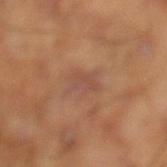{
  "biopsy_status": "not biopsied; imaged during a skin examination",
  "site": "left lower leg",
  "lesion_size": {
    "long_diameter_mm_approx": 4.0
  },
  "lighting": "cross-polarized",
  "patient": {
    "sex": "male",
    "age_approx": 45
  },
  "image": {
    "source": "total-body photography crop",
    "field_of_view_mm": 15
  },
  "automated_metrics": {
    "area_mm2_approx": 6.5,
    "eccentricity": 0.85,
    "shape_asymmetry": 0.3,
    "border_irregularity_0_10": 4.0,
    "color_variation_0_10": 3.0,
    "peripheral_color_asymmetry": 1.0
  }
}